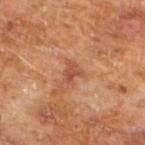{
  "biopsy_status": "not biopsied; imaged during a skin examination",
  "patient": {
    "sex": "male",
    "age_approx": 65
  },
  "image": {
    "source": "total-body photography crop",
    "field_of_view_mm": 15
  }
}